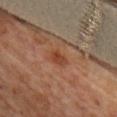{
  "biopsy_status": "not biopsied; imaged during a skin examination",
  "lesion_size": {
    "long_diameter_mm_approx": 2.5
  },
  "patient": {
    "sex": "female",
    "age_approx": 65
  },
  "automated_metrics": {
    "vs_skin_darker_L": 8.0,
    "vs_skin_contrast_norm": 7.0,
    "nevus_likeness_0_100": 65,
    "lesion_detection_confidence_0_100": 100
  },
  "lighting": "cross-polarized",
  "site": "back",
  "image": {
    "source": "total-body photography crop",
    "field_of_view_mm": 15
  }
}